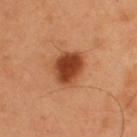Case summary:
– biopsy status · catalogued during a skin exam; not biopsied
– patient · male, aged approximately 55
– lesion size · ≈4 mm
– acquisition · total-body-photography crop, ~15 mm field of view
– tile lighting · cross-polarized illumination
– image-analysis metrics · a footprint of about 12 mm² and a symmetry-axis asymmetry near 0.2; a lesion color around L≈43 a*≈27 b*≈36 in CIELAB and about 15 CIELAB-L* units darker than the surrounding skin; border irregularity of about 2 on a 0–10 scale and radial color variation of about 1.5; a classifier nevus-likeness of about 100/100
– site · the upper back